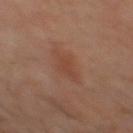- acquisition · ~15 mm crop, total-body skin-cancer survey
- patient · male, about 60 years old
- location · the mid back